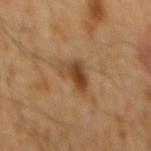The lesion was tiled from a total-body skin photograph and was not biopsied. Automated image analysis of the tile measured an area of roughly 6.5 mm², a shape eccentricity near 0.65, and a symmetry-axis asymmetry near 0.5. And it measured a mean CIELAB color near L≈39 a*≈18 b*≈32, about 10 CIELAB-L* units darker than the surrounding skin, and a lesion-to-skin contrast of about 8.5 (normalized; higher = more distinct). And it measured a nevus-likeness score of about 90/100 and lesion-presence confidence of about 100/100. Approximately 3.5 mm at its widest. On the mid back. Captured under cross-polarized illumination. A 15 mm close-up extracted from a 3D total-body photography capture. A male patient in their 60s.